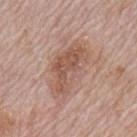Imaged during a routine full-body skin examination; the lesion was not biopsied and no histopathology is available.
The lesion-visualizer software estimated a mean CIELAB color near L≈55 a*≈20 b*≈27 and a normalized lesion–skin contrast near 7. The analysis additionally found a border-irregularity rating of about 3.5/10, a color-variation rating of about 5/10, and a peripheral color-asymmetry measure near 1.5. The analysis additionally found a classifier nevus-likeness of about 35/100.
Captured under white-light illumination.
Longest diameter approximately 6.5 mm.
A male subject approximately 70 years of age.
On the mid back.
A region of skin cropped from a whole-body photographic capture, roughly 15 mm wide.